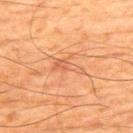workup = catalogued during a skin exam; not biopsied
lesion size = ~3.5 mm (longest diameter)
patient = male, aged 63 to 67
site = the upper back
image = ~15 mm tile from a whole-body skin photo